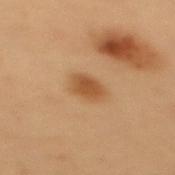Notes:
• notes: imaged on a skin check; not biopsied
• subject: female, aged around 40
• body site: the upper back
• automated lesion analysis: radial color variation of about 0.5; a lesion-detection confidence of about 100/100
• imaging modality: ~15 mm tile from a whole-body skin photo
• tile lighting: cross-polarized
• lesion diameter: ~3 mm (longest diameter)